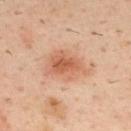follow-up: total-body-photography surveillance lesion; no biopsy
acquisition: ~15 mm tile from a whole-body skin photo
body site: the upper back
lesion size: about 5 mm
patient: male, in their 40s
image-analysis metrics: a footprint of about 12 mm², a shape eccentricity near 0.75, and a symmetry-axis asymmetry near 0.25; an average lesion color of about L≈59 a*≈24 b*≈33 (CIELAB) and a lesion-to-skin contrast of about 7 (normalized; higher = more distinct); lesion-presence confidence of about 100/100